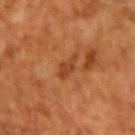Imaged during a routine full-body skin examination; the lesion was not biopsied and no histopathology is available. Captured under cross-polarized illumination. This image is a 15 mm lesion crop taken from a total-body photograph. From the right upper arm. A male subject roughly 70 years of age.